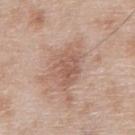biopsy status — total-body-photography surveillance lesion; no biopsy | acquisition — 15 mm crop, total-body photography | subject — male, approximately 80 years of age | anatomic site — the chest | automated metrics — a lesion area of about 10 mm², a shape eccentricity near 0.75, and two-axis asymmetry of about 0.25; a border-irregularity rating of about 4/10 and internal color variation of about 3 on a 0–10 scale; a nevus-likeness score of about 0/100 and a lesion-detection confidence of about 100/100 | tile lighting — white-light illumination.